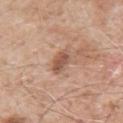{
  "biopsy_status": "not biopsied; imaged during a skin examination",
  "patient": {
    "sex": "male",
    "age_approx": 60
  },
  "lesion_size": {
    "long_diameter_mm_approx": 3.0
  },
  "site": "left upper arm",
  "image": {
    "source": "total-body photography crop",
    "field_of_view_mm": 15
  },
  "lighting": "white-light",
  "automated_metrics": {
    "cielab_L": 54,
    "cielab_a": 21,
    "cielab_b": 29,
    "vs_skin_darker_L": 11.0
  }
}